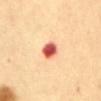workup = no biopsy performed (imaged during a skin exam) | automated lesion analysis = a lesion area of about 5 mm² and a shape eccentricity near 0.45; a classifier nevus-likeness of about 0/100 | image = ~15 mm tile from a whole-body skin photo | tile lighting = cross-polarized | patient = female, aged approximately 60 | lesion diameter = ≈2.5 mm | site = the abdomen.